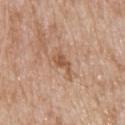{
  "biopsy_status": "not biopsied; imaged during a skin examination",
  "image": {
    "source": "total-body photography crop",
    "field_of_view_mm": 15
  },
  "site": "upper back",
  "lesion_size": {
    "long_diameter_mm_approx": 3.0
  },
  "patient": {
    "sex": "male",
    "age_approx": 80
  },
  "lighting": "white-light"
}